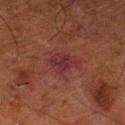<tbp_lesion>
  <biopsy_status>not biopsied; imaged during a skin examination</biopsy_status>
  <lighting>cross-polarized</lighting>
  <site>left lower leg</site>
  <image>
    <source>total-body photography crop</source>
    <field_of_view_mm>15</field_of_view_mm>
  </image>
  <lesion_size>
    <long_diameter_mm_approx>3.5</long_diameter_mm_approx>
  </lesion_size>
  <patient>
    <sex>male</sex>
    <age_approx>80</age_approx>
  </patient>
</tbp_lesion>